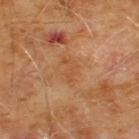Assessment: This lesion was catalogued during total-body skin photography and was not selected for biopsy. Clinical summary: A male subject, approximately 60 years of age. Measured at roughly 3 mm in maximum diameter. Imaged with cross-polarized lighting. From the chest. An algorithmic analysis of the crop reported a mean CIELAB color near L≈49 a*≈22 b*≈37, roughly 6 lightness units darker than nearby skin, and a normalized lesion–skin contrast near 5. A close-up tile cropped from a whole-body skin photograph, about 15 mm across.